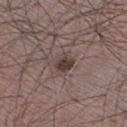biopsy status: imaged on a skin check; not biopsied
image source: ~15 mm tile from a whole-body skin photo
patient: male, aged around 35
lesion size: ≈3 mm
location: the right thigh
tile lighting: white-light